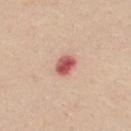Notes:
– workup — catalogued during a skin exam; not biopsied
– patient — female, approximately 40 years of age
– acquisition — total-body-photography crop, ~15 mm field of view
– site — the chest